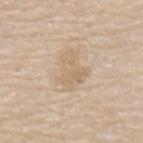The lesion was photographed on a routine skin check and not biopsied; there is no pathology result. On the mid back. Captured under white-light illumination. The patient is a male roughly 75 years of age. A 15 mm close-up extracted from a 3D total-body photography capture. Approximately 4.5 mm at its widest.